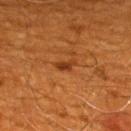About 2.5 mm across. Captured under cross-polarized illumination. Cropped from a whole-body photographic skin survey; the tile spans about 15 mm. An algorithmic analysis of the crop reported a footprint of about 2.5 mm² and two-axis asymmetry of about 0.45. The software also gave a lesion color around L≈36 a*≈27 b*≈38 in CIELAB and a normalized lesion–skin contrast near 8. The software also gave border irregularity of about 4 on a 0–10 scale, internal color variation of about 0.5 on a 0–10 scale, and a peripheral color-asymmetry measure near 0. From the mid back. The patient is a male roughly 60 years of age.